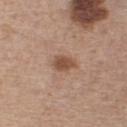{
  "biopsy_status": "not biopsied; imaged during a skin examination",
  "image": {
    "source": "total-body photography crop",
    "field_of_view_mm": 15
  },
  "site": "front of the torso",
  "lighting": "white-light",
  "patient": {
    "sex": "female",
    "age_approx": 45
  },
  "automated_metrics": {
    "area_mm2_approx": 5.0,
    "eccentricity": 0.75,
    "shape_asymmetry": 0.25,
    "cielab_L": 50,
    "cielab_a": 20,
    "cielab_b": 29,
    "vs_skin_contrast_norm": 8.0,
    "lesion_detection_confidence_0_100": 100
  },
  "lesion_size": {
    "long_diameter_mm_approx": 3.0
  }
}